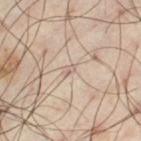imaging modality = ~15 mm crop, total-body skin-cancer survey
lighting = cross-polarized illumination
patient = male, about 40 years old
size = ~1.5 mm (longest diameter)
site = the left thigh
automated metrics = a footprint of about 1 mm² and a shape eccentricity near 0.9; a border-irregularity rating of about 3/10 and radial color variation of about 0; a classifier nevus-likeness of about 0/100 and lesion-presence confidence of about 0/100